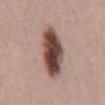A region of skin cropped from a whole-body photographic capture, roughly 15 mm wide. On the mid back. The recorded lesion diameter is about 7.5 mm. This is a white-light tile. A male patient about 45 years old.